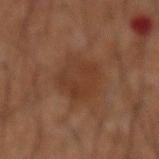Recorded during total-body skin imaging; not selected for excision or biopsy. Longest diameter approximately 6 mm. A 15 mm close-up extracted from a 3D total-body photography capture. The lesion is located on the mid back. Captured under cross-polarized illumination. The subject is a male approximately 60 years of age.